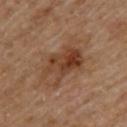Q: Was a biopsy performed?
A: imaged on a skin check; not biopsied
Q: How was this image acquired?
A: ~15 mm crop, total-body skin-cancer survey
Q: What did automated image analysis measure?
A: a lesion area of about 14 mm²
Q: Lesion location?
A: the upper back
Q: Lesion size?
A: about 6 mm
Q: Who is the patient?
A: male, aged around 85 or older
Q: What lighting was used for the tile?
A: cross-polarized illumination